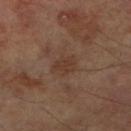Assessment:
The lesion was photographed on a routine skin check and not biopsied; there is no pathology result.
Clinical summary:
Automated image analysis of the tile measured a border-irregularity rating of about 2.5/10. The recorded lesion diameter is about 3 mm. The lesion is on the right lower leg. A 15 mm crop from a total-body photograph taken for skin-cancer surveillance. The patient is a male roughly 70 years of age. The tile uses cross-polarized illumination.